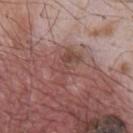The tile uses white-light illumination. Automated tile analysis of the lesion measured an automated nevus-likeness rating near 0 out of 100 and a lesion-detection confidence of about 90/100. A male patient aged around 65. Approximately 4.5 mm at its widest. On the chest. A 15 mm close-up tile from a total-body photography series done for melanoma screening.